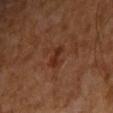Q: Is there a histopathology result?
A: total-body-photography surveillance lesion; no biopsy
Q: Illumination type?
A: cross-polarized
Q: How was this image acquired?
A: total-body-photography crop, ~15 mm field of view
Q: Who is the patient?
A: male, aged 63–67
Q: What is the anatomic site?
A: the right upper arm
Q: Lesion size?
A: ~2.5 mm (longest diameter)